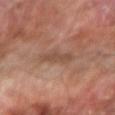Part of a total-body skin-imaging series; this lesion was reviewed on a skin check and was not flagged for biopsy. A male subject aged approximately 65. The lesion is on the right forearm. The recorded lesion diameter is about 3.5 mm. This is a cross-polarized tile. Cropped from a total-body skin-imaging series; the visible field is about 15 mm.